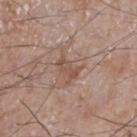notes: no biopsy performed (imaged during a skin exam)
illumination: white-light illumination
subject: male, about 75 years old
automated metrics: a lesion area of about 4 mm² and a shape-asymmetry score of about 0.3 (0 = symmetric); an average lesion color of about L≈51 a*≈18 b*≈27 (CIELAB), about 8 CIELAB-L* units darker than the surrounding skin, and a normalized lesion–skin contrast near 6; a border-irregularity rating of about 4/10, internal color variation of about 1 on a 0–10 scale, and a peripheral color-asymmetry measure near 0; lesion-presence confidence of about 100/100
anatomic site: the chest
image source: ~15 mm crop, total-body skin-cancer survey